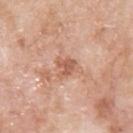Q: Was this lesion biopsied?
A: imaged on a skin check; not biopsied
Q: How was this image acquired?
A: total-body-photography crop, ~15 mm field of view
Q: How was the tile lit?
A: white-light
Q: Lesion location?
A: the back
Q: Who is the patient?
A: male, in their 80s